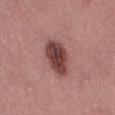Imaged during a routine full-body skin examination; the lesion was not biopsied and no histopathology is available. This image is a 15 mm lesion crop taken from a total-body photograph. The lesion is on the left thigh. A male patient, aged 53 to 57.